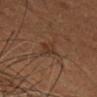TBP lesion metrics: a lesion area of about 3 mm², an outline eccentricity of about 0.9 (0 = round, 1 = elongated), and two-axis asymmetry of about 0.4; about 5 CIELAB-L* units darker than the surrounding skin and a normalized lesion–skin contrast near 6; border irregularity of about 4 on a 0–10 scale, internal color variation of about 1 on a 0–10 scale, and a peripheral color-asymmetry measure near 0.5 | imaging modality: ~15 mm tile from a whole-body skin photo | anatomic site: the head or neck | patient: male, in their mid-50s | lesion diameter: ≈3 mm.